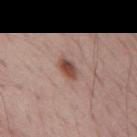Part of a total-body skin-imaging series; this lesion was reviewed on a skin check and was not flagged for biopsy.
The patient is a male aged around 70.
This image is a 15 mm lesion crop taken from a total-body photograph.
On the mid back.
Longest diameter approximately 3 mm.
The tile uses white-light illumination.